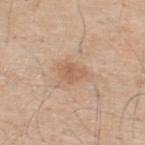notes: catalogued during a skin exam; not biopsied
subject: male, aged approximately 70
lesion size: about 2.5 mm
TBP lesion metrics: an area of roughly 4 mm², an outline eccentricity of about 0.65 (0 = round, 1 = elongated), and two-axis asymmetry of about 0.35; a mean CIELAB color near L≈60 a*≈18 b*≈32 and about 8 CIELAB-L* units darker than the surrounding skin; a border-irregularity rating of about 3/10, a within-lesion color-variation index near 2/10, and radial color variation of about 0.5; a nevus-likeness score of about 0/100 and lesion-presence confidence of about 100/100
image: ~15 mm crop, total-body skin-cancer survey
location: the back
tile lighting: white-light illumination